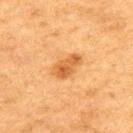The lesion was photographed on a routine skin check and not biopsied; there is no pathology result.
The recorded lesion diameter is about 4 mm.
A male patient, aged 73–77.
The lesion is located on the upper back.
A close-up tile cropped from a whole-body skin photograph, about 15 mm across.